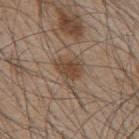A male subject about 45 years old.
On the back.
Longest diameter approximately 4 mm.
A 15 mm crop from a total-body photograph taken for skin-cancer surveillance.